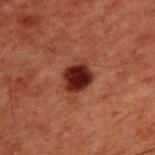Captured during whole-body skin photography for melanoma surveillance; the lesion was not biopsied. On the upper back. A male patient aged approximately 60. A 15 mm crop from a total-body photograph taken for skin-cancer surveillance. This is a cross-polarized tile. Longest diameter approximately 3.5 mm.